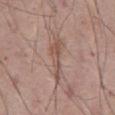Impression: Recorded during total-body skin imaging; not selected for excision or biopsy. Background: A male patient aged 53 to 57. Located on the abdomen. The tile uses white-light illumination. This image is a 15 mm lesion crop taken from a total-body photograph.